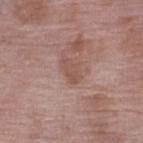{"biopsy_status": "not biopsied; imaged during a skin examination"}